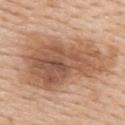Recorded during total-body skin imaging; not selected for excision or biopsy.
Automated tile analysis of the lesion measured a footprint of about 65 mm², a shape eccentricity near 0.85, and two-axis asymmetry of about 0.3. The analysis additionally found an average lesion color of about L≈58 a*≈20 b*≈32 (CIELAB), about 13 CIELAB-L* units darker than the surrounding skin, and a lesion-to-skin contrast of about 8.5 (normalized; higher = more distinct). The software also gave a border-irregularity index near 4.5/10 and a within-lesion color-variation index near 7/10. The analysis additionally found a nevus-likeness score of about 70/100 and a detector confidence of about 100 out of 100 that the crop contains a lesion.
Captured under white-light illumination.
A region of skin cropped from a whole-body photographic capture, roughly 15 mm wide.
Measured at roughly 13 mm in maximum diameter.
A female patient, in their mid-60s.
On the upper back.